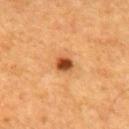Located on the back. This image is a 15 mm lesion crop taken from a total-body photograph. Longest diameter approximately 2 mm. The subject is a male aged 58 to 62. Automated image analysis of the tile measured a border-irregularity index near 1.5/10, a within-lesion color-variation index near 5/10, and radial color variation of about 1.5.